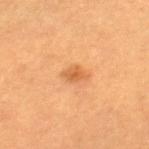The lesion's longest dimension is about 2.5 mm. The subject is a female about 30 years old. A 15 mm crop from a total-body photograph taken for skin-cancer surveillance. On the left leg. An algorithmic analysis of the crop reported a border-irregularity rating of about 2.5/10, a color-variation rating of about 2/10, and peripheral color asymmetry of about 0.5.